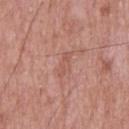Recorded during total-body skin imaging; not selected for excision or biopsy.
A male subject, about 55 years old.
Imaged with white-light lighting.
Automated image analysis of the tile measured a shape eccentricity near 0.95. And it measured a lesion color around L≈55 a*≈24 b*≈27 in CIELAB, a lesion–skin lightness drop of about 6, and a lesion-to-skin contrast of about 4.5 (normalized; higher = more distinct).
This image is a 15 mm lesion crop taken from a total-body photograph.
Measured at roughly 3 mm in maximum diameter.